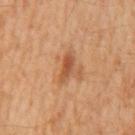Clinical impression:
Part of a total-body skin-imaging series; this lesion was reviewed on a skin check and was not flagged for biopsy.
Image and clinical context:
A 15 mm close-up extracted from a 3D total-body photography capture. This is a cross-polarized tile. A male subject, aged 58 to 62. The lesion's longest dimension is about 3.5 mm. On the mid back.